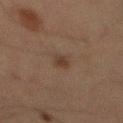Impression: The lesion was photographed on a routine skin check and not biopsied; there is no pathology result. Background: Located on the abdomen. The subject is a male approximately 65 years of age. A region of skin cropped from a whole-body photographic capture, roughly 15 mm wide.